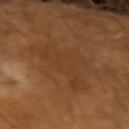biopsy status = catalogued during a skin exam; not biopsied | size = ~7 mm (longest diameter) | subject = male, in their mid-60s | illumination = cross-polarized | imaging modality = 15 mm crop, total-body photography.